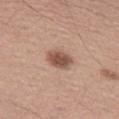Assessment: Part of a total-body skin-imaging series; this lesion was reviewed on a skin check and was not flagged for biopsy. Context: Located on the abdomen. The subject is a male aged 53–57. About 3.5 mm across. This is a white-light tile. The lesion-visualizer software estimated an area of roughly 7 mm², an outline eccentricity of about 0.75 (0 = round, 1 = elongated), and a symmetry-axis asymmetry near 0.15. It also reported a classifier nevus-likeness of about 95/100. Cropped from a total-body skin-imaging series; the visible field is about 15 mm.